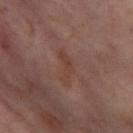Clinical impression: Imaged during a routine full-body skin examination; the lesion was not biopsied and no histopathology is available. Acquisition and patient details: Located on the right thigh. A region of skin cropped from a whole-body photographic capture, roughly 15 mm wide. Longest diameter approximately 4.5 mm. A female patient, in their mid-50s. Automated tile analysis of the lesion measured an area of roughly 5 mm² and an eccentricity of roughly 0.95.